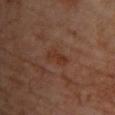This lesion was catalogued during total-body skin photography and was not selected for biopsy. A 15 mm crop from a total-body photograph taken for skin-cancer surveillance. The patient is a female approximately 65 years of age. The lesion is on the upper back.